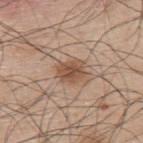{"image": {"source": "total-body photography crop", "field_of_view_mm": 15}, "patient": {"sex": "male", "age_approx": 45}, "site": "upper back"}